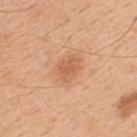follow-up=imaged on a skin check; not biopsied | subject=male, aged 48 to 52 | lesion diameter=~3 mm (longest diameter) | lighting=white-light | site=the upper back | imaging modality=15 mm crop, total-body photography.